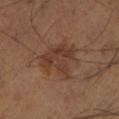{
  "biopsy_status": "not biopsied; imaged during a skin examination",
  "lesion_size": {
    "long_diameter_mm_approx": 5.0
  },
  "lighting": "cross-polarized",
  "image": {
    "source": "total-body photography crop",
    "field_of_view_mm": 15
  },
  "site": "left lower leg",
  "patient": {
    "sex": "male",
    "age_approx": 55
  }
}